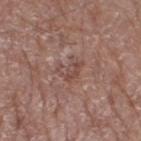workup=total-body-photography surveillance lesion; no biopsy
subject=female, approximately 75 years of age
anatomic site=the left lower leg
lighting=white-light
image source=15 mm crop, total-body photography